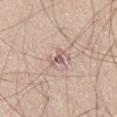A 15 mm close-up extracted from a 3D total-body photography capture.
Captured under white-light illumination.
About 3 mm across.
A male patient, aged 28–32.
On the right thigh.
An algorithmic analysis of the crop reported a footprint of about 2.5 mm², an outline eccentricity of about 0.9 (0 = round, 1 = elongated), and a symmetry-axis asymmetry near 0.6. The analysis additionally found a border-irregularity rating of about 6/10. And it measured lesion-presence confidence of about 85/100.